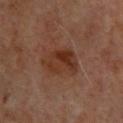Captured during whole-body skin photography for melanoma surveillance; the lesion was not biopsied. Located on the upper back. Longest diameter approximately 4.5 mm. Automated tile analysis of the lesion measured a footprint of about 11 mm² and a shape eccentricity near 0.75. It also reported a border-irregularity index near 2.5/10, a color-variation rating of about 6.5/10, and radial color variation of about 2.5. A male subject aged 63–67. A 15 mm close-up tile from a total-body photography series done for melanoma screening. The tile uses cross-polarized illumination.